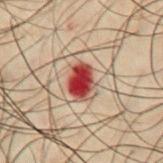Part of a total-body skin-imaging series; this lesion was reviewed on a skin check and was not flagged for biopsy. Cropped from a total-body skin-imaging series; the visible field is about 15 mm. Automated image analysis of the tile measured a mean CIELAB color near L≈34 a*≈29 b*≈24 and a lesion–skin lightness drop of about 17. It also reported a color-variation rating of about 4.5/10 and radial color variation of about 1.5. The lesion is located on the abdomen. Measured at roughly 4 mm in maximum diameter. A male patient, approximately 50 years of age.